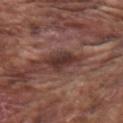<case>
<biopsy_status>not biopsied; imaged during a skin examination</biopsy_status>
<image>
  <source>total-body photography crop</source>
  <field_of_view_mm>15</field_of_view_mm>
</image>
<patient>
  <sex>male</sex>
  <age_approx>75</age_approx>
</patient>
<automated_metrics>
  <cielab_L>34</cielab_L>
  <cielab_a>20</cielab_a>
  <cielab_b>21</cielab_b>
  <vs_skin_darker_L>11.0</vs_skin_darker_L>
  <vs_skin_contrast_norm>10.0</vs_skin_contrast_norm>
  <border_irregularity_0_10>3.0</border_irregularity_0_10>
  <peripheral_color_asymmetry>1.0</peripheral_color_asymmetry>
  <nevus_likeness_0_100>20</nevus_likeness_0_100>
  <lesion_detection_confidence_0_100>85</lesion_detection_confidence_0_100>
</automated_metrics>
<site>arm</site>
<lesion_size>
  <long_diameter_mm_approx>4.0</long_diameter_mm_approx>
</lesion_size>
<lighting>white-light</lighting>
</case>